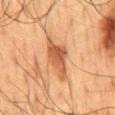* workup · no biopsy performed (imaged during a skin exam)
* patient · male, roughly 60 years of age
* tile lighting · cross-polarized
* acquisition · 15 mm crop, total-body photography
* diameter · ≈6 mm
* site · the mid back
* automated lesion analysis · a lesion color around L≈44 a*≈21 b*≈32 in CIELAB and roughly 10 lightness units darker than nearby skin; border irregularity of about 4 on a 0–10 scale and internal color variation of about 4 on a 0–10 scale